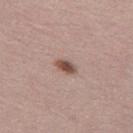• biopsy status: total-body-photography surveillance lesion; no biopsy
• acquisition: 15 mm crop, total-body photography
• subject: female, aged 53 to 57
• body site: the left thigh